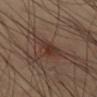Imaged during a routine full-body skin examination; the lesion was not biopsied and no histopathology is available. This image is a 15 mm lesion crop taken from a total-body photograph. From the left thigh. The recorded lesion diameter is about 5.5 mm. A male patient, about 40 years old. The lesion-visualizer software estimated a lesion color around L≈34 a*≈18 b*≈23 in CIELAB. The software also gave an automated nevus-likeness rating near 5 out of 100 and lesion-presence confidence of about 95/100.